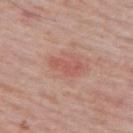Impression:
Recorded during total-body skin imaging; not selected for excision or biopsy.
Context:
On the upper back. An algorithmic analysis of the crop reported an area of roughly 5 mm², an eccentricity of roughly 0.9, and a shape-asymmetry score of about 0.5 (0 = symmetric). It also reported a classifier nevus-likeness of about 15/100 and a lesion-detection confidence of about 100/100. A roughly 15 mm field-of-view crop from a total-body skin photograph. The subject is a male approximately 65 years of age. The recorded lesion diameter is about 4 mm.